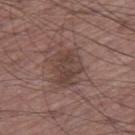No biopsy was performed on this lesion — it was imaged during a full skin examination and was not determined to be concerning.
Imaged with white-light lighting.
Cropped from a total-body skin-imaging series; the visible field is about 15 mm.
A male patient about 60 years old.
From the right thigh.